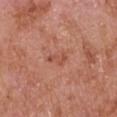Measured at roughly 3 mm in maximum diameter.
The subject is a male roughly 65 years of age.
This image is a 15 mm lesion crop taken from a total-body photograph.
Automated image analysis of the tile measured a lesion–skin lightness drop of about 7 and a normalized lesion–skin contrast near 5.5. The software also gave border irregularity of about 6 on a 0–10 scale and internal color variation of about 0 on a 0–10 scale.
The tile uses white-light illumination.
On the left upper arm.